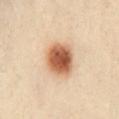* diameter: about 4.5 mm
* subject: female, in their 40s
* automated metrics: a lesion area of about 12 mm², an eccentricity of roughly 0.65, and a shape-asymmetry score of about 0.1 (0 = symmetric); a mean CIELAB color near L≈60 a*≈24 b*≈36, a lesion–skin lightness drop of about 20, and a normalized border contrast of about 12; a border-irregularity rating of about 1/10, a within-lesion color-variation index near 6.5/10, and radial color variation of about 2; an automated nevus-likeness rating near 100 out of 100 and a detector confidence of about 100 out of 100 that the crop contains a lesion
* body site: the chest
* illumination: cross-polarized illumination
* image: ~15 mm tile from a whole-body skin photo